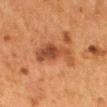Part of a total-body skin-imaging series; this lesion was reviewed on a skin check and was not flagged for biopsy. About 6 mm across. A roughly 15 mm field-of-view crop from a total-body skin photograph. Imaged with cross-polarized lighting. A female subject, aged 48 to 52. The lesion is on the mid back.